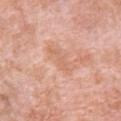notes: imaged on a skin check; not biopsied | lighting: white-light | automated metrics: a lesion area of about 4 mm², an outline eccentricity of about 0.9 (0 = round, 1 = elongated), and a shape-asymmetry score of about 0.35 (0 = symmetric); border irregularity of about 5 on a 0–10 scale, a within-lesion color-variation index near 0.5/10, and radial color variation of about 0 | body site: the chest | subject: male, about 50 years old | acquisition: ~15 mm tile from a whole-body skin photo | lesion diameter: ~3.5 mm (longest diameter).